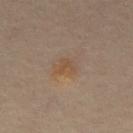workup=imaged on a skin check; not biopsied | subject=male, aged 48–52 | anatomic site=the abdomen | acquisition=~15 mm crop, total-body skin-cancer survey.